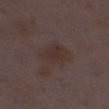Imaged during a routine full-body skin examination; the lesion was not biopsied and no histopathology is available. A 15 mm close-up tile from a total-body photography series done for melanoma screening. The patient is a female approximately 30 years of age. This is a white-light tile. The lesion is on the left lower leg. About 3 mm across.